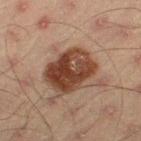<case>
<biopsy_status>not biopsied; imaged during a skin examination</biopsy_status>
<automated_metrics>
  <cielab_L>33</cielab_L>
  <cielab_a>18</cielab_a>
  <cielab_b>25</cielab_b>
  <vs_skin_darker_L>13.0</vs_skin_darker_L>
  <vs_skin_contrast_norm>12.0</vs_skin_contrast_norm>
  <border_irregularity_0_10>1.5</border_irregularity_0_10>
  <color_variation_0_10>7.5</color_variation_0_10>
  <peripheral_color_asymmetry>2.5</peripheral_color_asymmetry>
</automated_metrics>
<image>
  <source>total-body photography crop</source>
  <field_of_view_mm>15</field_of_view_mm>
</image>
<patient>
  <sex>male</sex>
  <age_approx>45</age_approx>
</patient>
<lighting>cross-polarized</lighting>
<lesion_size>
  <long_diameter_mm_approx>5.5</long_diameter_mm_approx>
</lesion_size>
<site>right thigh</site>
</case>